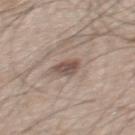Notes:
– acquisition — ~15 mm tile from a whole-body skin photo
– lighting — white-light illumination
– automated metrics — a footprint of about 4.5 mm², an outline eccentricity of about 0.6 (0 = round, 1 = elongated), and a symmetry-axis asymmetry near 0.25; a lesion color around L≈50 a*≈15 b*≈22 in CIELAB, roughly 12 lightness units darker than nearby skin, and a normalized lesion–skin contrast near 8.5; a border-irregularity rating of about 2/10, internal color variation of about 4 on a 0–10 scale, and peripheral color asymmetry of about 1.5
– body site — the back
– subject — male, roughly 50 years of age